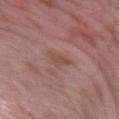| key | value |
|---|---|
| biopsy status | no biopsy performed (imaged during a skin exam) |
| image-analysis metrics | a lesion area of about 4 mm² and a shape eccentricity near 0.75; an average lesion color of about L≈49 a*≈20 b*≈24 (CIELAB) and a lesion–skin lightness drop of about 6; border irregularity of about 3.5 on a 0–10 scale and internal color variation of about 1.5 on a 0–10 scale |
| anatomic site | the arm |
| patient | male, approximately 40 years of age |
| image source | ~15 mm tile from a whole-body skin photo |